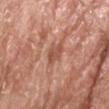workup: no biopsy performed (imaged during a skin exam)
size: ~3.5 mm (longest diameter)
image: total-body-photography crop, ~15 mm field of view
site: the head or neck
subject: male, aged approximately 65
tile lighting: white-light